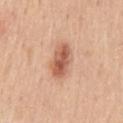Q: Was a biopsy performed?
A: imaged on a skin check; not biopsied
Q: What are the patient's age and sex?
A: male, in their 50s
Q: What did automated image analysis measure?
A: roughly 14 lightness units darker than nearby skin and a normalized lesion–skin contrast near 8.5; a nevus-likeness score of about 95/100 and lesion-presence confidence of about 100/100
Q: What lighting was used for the tile?
A: white-light illumination
Q: What is the imaging modality?
A: ~15 mm crop, total-body skin-cancer survey
Q: What is the lesion's diameter?
A: ~4.5 mm (longest diameter)
Q: Where on the body is the lesion?
A: the mid back